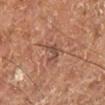biopsy status=no biopsy performed (imaged during a skin exam)
lighting=cross-polarized illumination
subject=male, aged 58 to 62
body site=the right leg
automated metrics=a shape eccentricity near 0.75 and a symmetry-axis asymmetry near 0.5; border irregularity of about 6 on a 0–10 scale, a color-variation rating of about 0/10, and a peripheral color-asymmetry measure near 0
acquisition=15 mm crop, total-body photography